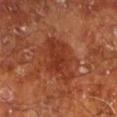follow-up: total-body-photography surveillance lesion; no biopsy
image-analysis metrics: an area of roughly 16 mm², an eccentricity of roughly 0.75, and a symmetry-axis asymmetry near 0.25; a mean CIELAB color near L≈35 a*≈29 b*≈32, about 8 CIELAB-L* units darker than the surrounding skin, and a normalized lesion–skin contrast near 7; a border-irregularity index near 3.5/10 and internal color variation of about 3 on a 0–10 scale
lesion diameter: ~5.5 mm (longest diameter)
body site: the left lower leg
subject: male, in their mid- to late 60s
lighting: cross-polarized illumination
image source: ~15 mm crop, total-body skin-cancer survey